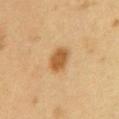No biopsy was performed on this lesion — it was imaged during a full skin examination and was not determined to be concerning.
A 15 mm close-up tile from a total-body photography series done for melanoma screening.
On the arm.
A female subject aged 28 to 32.